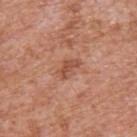| key | value |
|---|---|
| workup | catalogued during a skin exam; not biopsied |
| automated metrics | internal color variation of about 2.5 on a 0–10 scale and a peripheral color-asymmetry measure near 1; an automated nevus-likeness rating near 0 out of 100 and a detector confidence of about 100 out of 100 that the crop contains a lesion |
| subject | male, roughly 65 years of age |
| lesion diameter | ~3 mm (longest diameter) |
| lighting | white-light |
| image source | ~15 mm tile from a whole-body skin photo |
| anatomic site | the upper back |